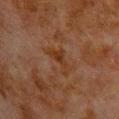Part of a total-body skin-imaging series; this lesion was reviewed on a skin check and was not flagged for biopsy.
This is a cross-polarized tile.
A lesion tile, about 15 mm wide, cut from a 3D total-body photograph.
Longest diameter approximately 3.5 mm.
The patient is a male in their 80s.
On the upper back.